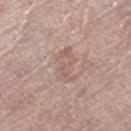Assessment: Captured during whole-body skin photography for melanoma surveillance; the lesion was not biopsied. Background: About 5 mm across. The tile uses white-light illumination. Automated tile analysis of the lesion measured a footprint of about 8.5 mm², a shape eccentricity near 0.9, and a symmetry-axis asymmetry near 0.3. The analysis additionally found a border-irregularity rating of about 4.5/10, a within-lesion color-variation index near 2.5/10, and peripheral color asymmetry of about 1. The subject is a female in their mid-60s. A 15 mm crop from a total-body photograph taken for skin-cancer surveillance. On the leg.